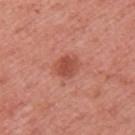Context:
Imaged with white-light lighting. The lesion is on the left upper arm. A lesion tile, about 15 mm wide, cut from a 3D total-body photograph. The subject is a female aged around 50.